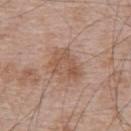notes = no biopsy performed (imaged during a skin exam) | illumination = white-light illumination | body site = the upper back | image source = 15 mm crop, total-body photography | image-analysis metrics = about 8 CIELAB-L* units darker than the surrounding skin and a normalized border contrast of about 6; a color-variation rating of about 3.5/10 and a peripheral color-asymmetry measure near 1; a nevus-likeness score of about 0/100 | patient = male, in their mid-60s | lesion diameter = ≈4.5 mm.